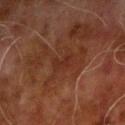| key | value |
|---|---|
| follow-up | catalogued during a skin exam; not biopsied |
| diameter | ~3 mm (longest diameter) |
| lighting | cross-polarized |
| automated lesion analysis | a lesion color around L≈24 a*≈20 b*≈24 in CIELAB, a lesion–skin lightness drop of about 4, and a normalized lesion–skin contrast near 4.5; a color-variation rating of about 2/10 and peripheral color asymmetry of about 0.5; a classifier nevus-likeness of about 0/100 |
| body site | the right upper arm |
| patient | male, roughly 70 years of age |
| image | total-body-photography crop, ~15 mm field of view |